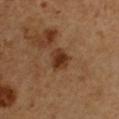Notes:
- follow-up · imaged on a skin check; not biopsied
- acquisition · ~15 mm crop, total-body skin-cancer survey
- body site · the arm
- subject · male, approximately 50 years of age
- image-analysis metrics · a lesion area of about 6 mm², an eccentricity of roughly 0.5, and two-axis asymmetry of about 0.2; an average lesion color of about L≈34 a*≈20 b*≈32 (CIELAB), about 10 CIELAB-L* units darker than the surrounding skin, and a normalized border contrast of about 9.5; a classifier nevus-likeness of about 75/100